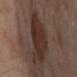Q: Is there a histopathology result?
A: no biopsy performed (imaged during a skin exam)
Q: What is the anatomic site?
A: the left lower leg
Q: What are the patient's age and sex?
A: female, aged 53 to 57
Q: Automated lesion metrics?
A: a footprint of about 26 mm², an outline eccentricity of about 0.85 (0 = round, 1 = elongated), and a symmetry-axis asymmetry near 0.3
Q: What kind of image is this?
A: ~15 mm tile from a whole-body skin photo